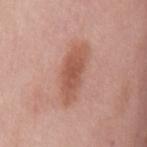Clinical impression: Imaged during a routine full-body skin examination; the lesion was not biopsied and no histopathology is available. Background: Longest diameter approximately 6.5 mm. The patient is a female about 60 years old. This is a white-light tile. Cropped from a whole-body photographic skin survey; the tile spans about 15 mm. An algorithmic analysis of the crop reported a lesion area of about 13 mm² and an eccentricity of roughly 0.95. And it measured a lesion color around L≈55 a*≈24 b*≈29 in CIELAB, a lesion–skin lightness drop of about 10, and a normalized lesion–skin contrast near 7.5. It also reported a classifier nevus-likeness of about 85/100 and a lesion-detection confidence of about 100/100. The lesion is located on the mid back.